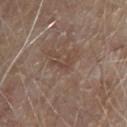No biopsy was performed on this lesion — it was imaged during a full skin examination and was not determined to be concerning. Longest diameter approximately 3 mm. On the chest. Automated tile analysis of the lesion measured an area of roughly 2.5 mm², an outline eccentricity of about 0.9 (0 = round, 1 = elongated), and two-axis asymmetry of about 0.7. The analysis additionally found an average lesion color of about L≈44 a*≈16 b*≈25 (CIELAB) and a lesion–skin lightness drop of about 6. A close-up tile cropped from a whole-body skin photograph, about 15 mm across. This is a white-light tile. The patient is a male aged approximately 80.